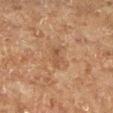notes — imaged on a skin check; not biopsied | body site — the left lower leg | patient — male, approximately 75 years of age | acquisition — ~15 mm crop, total-body skin-cancer survey | tile lighting — cross-polarized | lesion size — ≈3 mm.